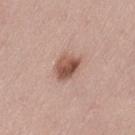Impression:
Recorded during total-body skin imaging; not selected for excision or biopsy.
Image and clinical context:
Imaged with white-light lighting. This image is a 15 mm lesion crop taken from a total-body photograph. From the left thigh. The patient is a female about 50 years old.